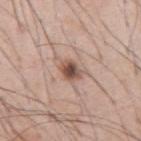tile lighting — white-light illumination
lesion size — ≈3 mm
image — ~15 mm tile from a whole-body skin photo
subject — male, approximately 55 years of age